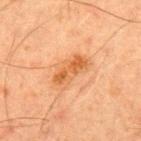The lesion was photographed on a routine skin check and not biopsied; there is no pathology result.
Automated image analysis of the tile measured a lesion area of about 8.5 mm² and a shape eccentricity near 0.9. The software also gave a color-variation rating of about 5/10 and a peripheral color-asymmetry measure near 1.5. And it measured a classifier nevus-likeness of about 30/100 and lesion-presence confidence of about 100/100.
A lesion tile, about 15 mm wide, cut from a 3D total-body photograph.
This is a cross-polarized tile.
The subject is a male approximately 45 years of age.
About 4.5 mm across.
From the upper back.